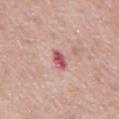Assessment: Recorded during total-body skin imaging; not selected for excision or biopsy. Context: A male subject, aged 73–77. The total-body-photography lesion software estimated a lesion area of about 3.5 mm², an eccentricity of roughly 0.8, and two-axis asymmetry of about 0.35. Measured at roughly 2.5 mm in maximum diameter. A close-up tile cropped from a whole-body skin photograph, about 15 mm across. The lesion is located on the back.